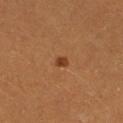<case>
  <biopsy_status>not biopsied; imaged during a skin examination</biopsy_status>
  <site>left leg</site>
  <lesion_size>
    <long_diameter_mm_approx>1.5</long_diameter_mm_approx>
  </lesion_size>
  <image>
    <source>total-body photography crop</source>
    <field_of_view_mm>15</field_of_view_mm>
  </image>
  <patient>
    <sex>female</sex>
    <age_approx>30</age_approx>
  </patient>
  <lighting>cross-polarized</lighting>
</case>